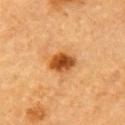| feature | finding |
|---|---|
| workup | catalogued during a skin exam; not biopsied |
| tile lighting | cross-polarized |
| lesion diameter | about 3.5 mm |
| acquisition | 15 mm crop, total-body photography |
| anatomic site | the right upper arm |
| automated lesion analysis | a lesion color around L≈44 a*≈25 b*≈41 in CIELAB, a lesion–skin lightness drop of about 15, and a lesion-to-skin contrast of about 11 (normalized; higher = more distinct) |
| patient | male, in their mid- to late 80s or older |